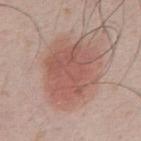biopsy_status: not biopsied; imaged during a skin examination
patient:
  sex: male
  age_approx: 50
image:
  source: total-body photography crop
  field_of_view_mm: 15
site: chest
automated_metrics:
  area_mm2_approx: 31.0
  shape_asymmetry: 0.2
  cielab_L: 55
  cielab_a: 22
  cielab_b: 25
  vs_skin_darker_L: 9.0
  vs_skin_contrast_norm: 6.5
  nevus_likeness_0_100: 95